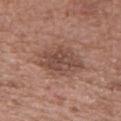Q: Was this lesion biopsied?
A: total-body-photography surveillance lesion; no biopsy
Q: Automated lesion metrics?
A: a shape eccentricity near 0.65 and two-axis asymmetry of about 0.15; a mean CIELAB color near L≈48 a*≈20 b*≈25, roughly 9 lightness units darker than nearby skin, and a lesion-to-skin contrast of about 7 (normalized; higher = more distinct); a peripheral color-asymmetry measure near 1
Q: Illumination type?
A: white-light illumination
Q: What is the imaging modality?
A: total-body-photography crop, ~15 mm field of view
Q: What is the anatomic site?
A: the mid back
Q: Who is the patient?
A: male, roughly 50 years of age
Q: How large is the lesion?
A: about 6 mm